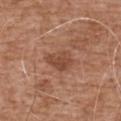Impression: Imaged during a routine full-body skin examination; the lesion was not biopsied and no histopathology is available. Acquisition and patient details: A male patient in their mid-70s. Located on the chest. The lesion's longest dimension is about 3.5 mm. Cropped from a total-body skin-imaging series; the visible field is about 15 mm. The lesion-visualizer software estimated a footprint of about 6.5 mm², a shape eccentricity near 0.65, and a symmetry-axis asymmetry near 0.25. The software also gave border irregularity of about 2.5 on a 0–10 scale and a peripheral color-asymmetry measure near 1. Imaged with white-light lighting.